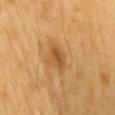| key | value |
|---|---|
| notes | imaged on a skin check; not biopsied |
| site | the mid back |
| subject | male, roughly 60 years of age |
| lesion diameter | ≈3.5 mm |
| tile lighting | cross-polarized illumination |
| imaging modality | 15 mm crop, total-body photography |
| image-analysis metrics | an eccentricity of roughly 0.85 and two-axis asymmetry of about 0.3; a nevus-likeness score of about 80/100 and lesion-presence confidence of about 100/100 |